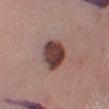  biopsy_status: not biopsied; imaged during a skin examination
  patient:
    sex: male
    age_approx: 65
  lesion_size:
    long_diameter_mm_approx: 4.0
  lighting: white-light
  image:
    source: total-body photography crop
    field_of_view_mm: 15
  site: left lower leg